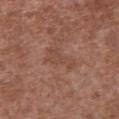Findings:
- follow-up — imaged on a skin check; not biopsied
- site — the chest
- image — 15 mm crop, total-body photography
- subject — male, aged approximately 45